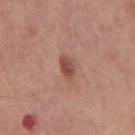Captured during whole-body skin photography for melanoma surveillance; the lesion was not biopsied. Automated tile analysis of the lesion measured a shape-asymmetry score of about 0.25 (0 = symmetric). The software also gave a lesion color around L≈49 a*≈24 b*≈28 in CIELAB, a lesion–skin lightness drop of about 10, and a lesion-to-skin contrast of about 7.5 (normalized; higher = more distinct). The software also gave a classifier nevus-likeness of about 90/100 and a detector confidence of about 100 out of 100 that the crop contains a lesion. This is a white-light tile. Cropped from a whole-body photographic skin survey; the tile spans about 15 mm. Longest diameter approximately 2.5 mm. The lesion is located on the mid back. The patient is a male approximately 60 years of age.